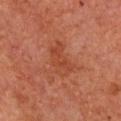workup = catalogued during a skin exam; not biopsied | subject = male, approximately 65 years of age | automated metrics = a lesion area of about 8.5 mm², a shape eccentricity near 0.85, and a symmetry-axis asymmetry near 0.35; border irregularity of about 4 on a 0–10 scale, a within-lesion color-variation index near 2.5/10, and peripheral color asymmetry of about 1; an automated nevus-likeness rating near 0 out of 100 and lesion-presence confidence of about 100/100 | site = the front of the torso | tile lighting = cross-polarized | imaging modality = total-body-photography crop, ~15 mm field of view.